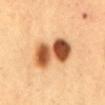The lesion was photographed on a routine skin check and not biopsied; there is no pathology result. The lesion's longest dimension is about 6 mm. The tile uses cross-polarized illumination. Automated tile analysis of the lesion measured an eccentricity of roughly 0.8 and a shape-asymmetry score of about 0.15 (0 = symmetric). The software also gave an average lesion color of about L≈56 a*≈25 b*≈40 (CIELAB), roughly 21 lightness units darker than nearby skin, and a lesion-to-skin contrast of about 12.5 (normalized; higher = more distinct). The lesion is located on the abdomen. The subject is a male in their 50s. A 15 mm close-up tile from a total-body photography series done for melanoma screening.